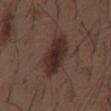Case summary:
* workup · no biopsy performed (imaged during a skin exam)
* automated lesion analysis · a footprint of about 12 mm², an outline eccentricity of about 0.9 (0 = round, 1 = elongated), and a shape-asymmetry score of about 0.2 (0 = symmetric)
* subject · male, aged 48–52
* body site · the mid back
* lighting · white-light illumination
* size · ~6 mm (longest diameter)
* image source · ~15 mm crop, total-body skin-cancer survey